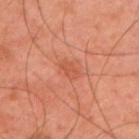<lesion>
<biopsy_status>not biopsied; imaged during a skin examination</biopsy_status>
<lesion_size>
  <long_diameter_mm_approx>2.5</long_diameter_mm_approx>
</lesion_size>
<image>
  <source>total-body photography crop</source>
  <field_of_view_mm>15</field_of_view_mm>
</image>
<automated_metrics>
  <cielab_L>51</cielab_L>
  <cielab_a>29</cielab_a>
  <cielab_b>34</cielab_b>
  <vs_skin_darker_L>6.0</vs_skin_darker_L>
  <vs_skin_contrast_norm>5.0</vs_skin_contrast_norm>
</automated_metrics>
<lighting>cross-polarized</lighting>
<patient>
  <sex>male</sex>
  <age_approx>45</age_approx>
</patient>
<site>right upper arm</site>
</lesion>